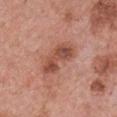- follow-up · total-body-photography surveillance lesion; no biopsy
- lighting · white-light
- patient · female, aged around 60
- lesion size · ≈5.5 mm
- image source · total-body-photography crop, ~15 mm field of view
- body site · the front of the torso
- image-analysis metrics · a lesion area of about 10 mm², a shape eccentricity near 0.9, and a shape-asymmetry score of about 0.35 (0 = symmetric); a mean CIELAB color near L≈50 a*≈25 b*≈29, a lesion–skin lightness drop of about 11, and a normalized border contrast of about 8; a border-irregularity rating of about 4/10, internal color variation of about 5.5 on a 0–10 scale, and a peripheral color-asymmetry measure near 1.5